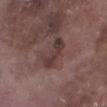{"biopsy_status": "not biopsied; imaged during a skin examination", "lighting": "white-light", "patient": {"sex": "male", "age_approx": 75}, "image": {"source": "total-body photography crop", "field_of_view_mm": 15}, "site": "right lower leg"}